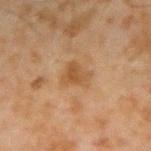Impression: Recorded during total-body skin imaging; not selected for excision or biopsy. Context: Measured at roughly 3.5 mm in maximum diameter. A male patient, aged around 45. This is a cross-polarized tile. A 15 mm close-up extracted from a 3D total-body photography capture. On the right forearm. Automated image analysis of the tile measured an area of roughly 6.5 mm², an eccentricity of roughly 0.65, and two-axis asymmetry of about 0.3. It also reported a lesion color around L≈42 a*≈17 b*≈32 in CIELAB, about 7 CIELAB-L* units darker than the surrounding skin, and a lesion-to-skin contrast of about 6.5 (normalized; higher = more distinct). The analysis additionally found lesion-presence confidence of about 100/100.